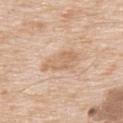Assessment:
Part of a total-body skin-imaging series; this lesion was reviewed on a skin check and was not flagged for biopsy.
Background:
From the upper back. The patient is a male in their mid-60s. A 15 mm close-up extracted from a 3D total-body photography capture.